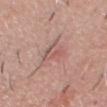The lesion was photographed on a routine skin check and not biopsied; there is no pathology result. Automated tile analysis of the lesion measured a footprint of about 7.5 mm², an eccentricity of roughly 0.7, and a symmetry-axis asymmetry near 0.4. And it measured a lesion color around L≈57 a*≈21 b*≈23 in CIELAB, roughly 7 lightness units darker than nearby skin, and a normalized border contrast of about 5. The analysis additionally found a within-lesion color-variation index near 5/10 and peripheral color asymmetry of about 2. A 15 mm close-up extracted from a 3D total-body photography capture. Approximately 4 mm at its widest. From the head or neck. A male subject roughly 50 years of age.